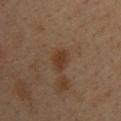The lesion was tiled from a total-body skin photograph and was not biopsied. This is a cross-polarized tile. A male subject, approximately 35 years of age. Located on the back. A 15 mm close-up tile from a total-body photography series done for melanoma screening. Measured at roughly 3 mm in maximum diameter.